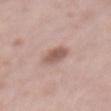Clinical impression:
The lesion was photographed on a routine skin check and not biopsied; there is no pathology result.
Clinical summary:
Captured under white-light illumination. The lesion is located on the mid back. Longest diameter approximately 4 mm. Cropped from a whole-body photographic skin survey; the tile spans about 15 mm. A male patient, aged approximately 75.